A male subject, approximately 60 years of age; the lesion is located on the head or neck; a 15 mm crop from a total-body photograph taken for skin-cancer surveillance.
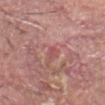Case summary:
– size: about 2 mm
– tile lighting: white-light
– histopathologic diagnosis: a squamous cell carcinoma in situ (malignant)